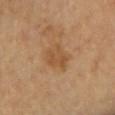{"biopsy_status": "not biopsied; imaged during a skin examination", "image": {"source": "total-body photography crop", "field_of_view_mm": 15}, "lesion_size": {"long_diameter_mm_approx": 3.5}, "patient": {"sex": "female", "age_approx": 60}, "automated_metrics": {"cielab_L": 52, "cielab_a": 20, "cielab_b": 38, "vs_skin_darker_L": 7.0, "nevus_likeness_0_100": 15, "lesion_detection_confidence_0_100": 100}, "site": "chest", "lighting": "cross-polarized"}